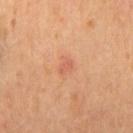acquisition = ~15 mm tile from a whole-body skin photo | tile lighting = cross-polarized | diameter = ≈2 mm | automated metrics = an eccentricity of roughly 0.8 and two-axis asymmetry of about 0.3; an automated nevus-likeness rating near 0 out of 100 and a lesion-detection confidence of about 100/100 | patient = male, aged approximately 55 | site = the front of the torso.